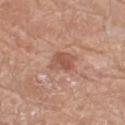Assessment: This lesion was catalogued during total-body skin photography and was not selected for biopsy. Acquisition and patient details: A female subject, roughly 75 years of age. Longest diameter approximately 3 mm. Automated tile analysis of the lesion measured a lesion area of about 6 mm², an eccentricity of roughly 0.6, and a shape-asymmetry score of about 0.3 (0 = symmetric). And it measured a normalized border contrast of about 6.5. The lesion is located on the right thigh. Captured under white-light illumination. A 15 mm close-up extracted from a 3D total-body photography capture.